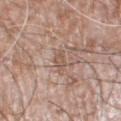Impression:
The lesion was photographed on a routine skin check and not biopsied; there is no pathology result.
Image and clinical context:
A male subject, aged 58–62. About 3 mm across. A 15 mm crop from a total-body photograph taken for skin-cancer surveillance. On the leg.